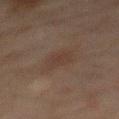Imaged during a routine full-body skin examination; the lesion was not biopsied and no histopathology is available.
Longest diameter approximately 3 mm.
A close-up tile cropped from a whole-body skin photograph, about 15 mm across.
A male patient, in their mid- to late 40s.
From the mid back.
Captured under cross-polarized illumination.